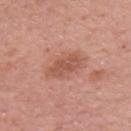Impression:
The lesion was tiled from a total-body skin photograph and was not biopsied.
Image and clinical context:
Measured at roughly 5 mm in maximum diameter. The tile uses white-light illumination. Located on the right upper arm. Automated tile analysis of the lesion measured a lesion area of about 10 mm², an eccentricity of roughly 0.8, and a shape-asymmetry score of about 0.2 (0 = symmetric). It also reported a border-irregularity rating of about 2.5/10, a color-variation rating of about 3/10, and radial color variation of about 1. The software also gave a detector confidence of about 100 out of 100 that the crop contains a lesion. Cropped from a whole-body photographic skin survey; the tile spans about 15 mm. The patient is a female aged around 50.